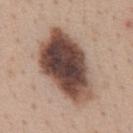| field | value |
|---|---|
| workup | catalogued during a skin exam; not biopsied |
| location | the front of the torso |
| patient | female, in their mid-40s |
| image | total-body-photography crop, ~15 mm field of view |
| lighting | white-light illumination |